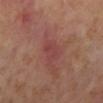The lesion was photographed on a routine skin check and not biopsied; there is no pathology result. Captured under cross-polarized illumination. This image is a 15 mm lesion crop taken from a total-body photograph. From the left lower leg. A female subject aged 53–57. Automated image analysis of the tile measured a footprint of about 4.5 mm² and a symmetry-axis asymmetry near 0.2. And it measured an average lesion color of about L≈43 a*≈27 b*≈23 (CIELAB), a lesion–skin lightness drop of about 6, and a lesion-to-skin contrast of about 5 (normalized; higher = more distinct). And it measured a lesion-detection confidence of about 95/100. About 3 mm across.